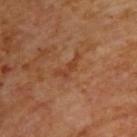The lesion was tiled from a total-body skin photograph and was not biopsied. Approximately 4 mm at its widest. A 15 mm close-up extracted from a 3D total-body photography capture. The total-body-photography lesion software estimated an area of roughly 4 mm², a shape eccentricity near 0.9, and a shape-asymmetry score of about 0.55 (0 = symmetric). It also reported an average lesion color of about L≈41 a*≈24 b*≈33 (CIELAB). And it measured a border-irregularity index near 6.5/10, internal color variation of about 0 on a 0–10 scale, and radial color variation of about 0. The analysis additionally found an automated nevus-likeness rating near 0 out of 100 and a detector confidence of about 95 out of 100 that the crop contains a lesion. A male patient aged around 65. On the back.